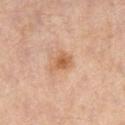biopsy status=no biopsy performed (imaged during a skin exam); location=the leg; subject=male, in their 50s; image source=~15 mm crop, total-body skin-cancer survey.